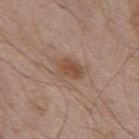Clinical impression: No biopsy was performed on this lesion — it was imaged during a full skin examination and was not determined to be concerning. Background: A male subject, aged 68 to 72. Captured under white-light illumination. A 15 mm close-up tile from a total-body photography series done for melanoma screening. Automated tile analysis of the lesion measured a lesion color around L≈50 a*≈18 b*≈28 in CIELAB and roughly 9 lightness units darker than nearby skin. The software also gave a border-irregularity rating of about 3.5/10 and radial color variation of about 1. The analysis additionally found a nevus-likeness score of about 90/100 and lesion-presence confidence of about 100/100. The recorded lesion diameter is about 4 mm. The lesion is located on the back.